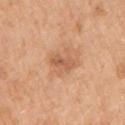Assessment: The lesion was photographed on a routine skin check and not biopsied; there is no pathology result. Clinical summary: The lesion's longest dimension is about 3.5 mm. A close-up tile cropped from a whole-body skin photograph, about 15 mm across. A female patient aged approximately 55. The tile uses white-light illumination. On the arm. The lesion-visualizer software estimated an area of roughly 4.5 mm² and an eccentricity of roughly 0.9. And it measured an average lesion color of about L≈59 a*≈24 b*≈35 (CIELAB), about 9 CIELAB-L* units darker than the surrounding skin, and a normalized lesion–skin contrast near 6. It also reported a border-irregularity index near 3/10 and radial color variation of about 1.5. It also reported a nevus-likeness score of about 0/100 and a lesion-detection confidence of about 100/100.